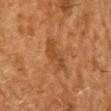The lesion was tiled from a total-body skin photograph and was not biopsied. Approximately 4.5 mm at its widest. A male patient aged around 70. The lesion is located on the head or neck. A 15 mm crop from a total-body photograph taken for skin-cancer surveillance. The tile uses cross-polarized illumination. Automated image analysis of the tile measured a lesion area of about 8.5 mm², an eccentricity of roughly 0.9, and a symmetry-axis asymmetry near 0.2. The software also gave border irregularity of about 3 on a 0–10 scale, internal color variation of about 3 on a 0–10 scale, and peripheral color asymmetry of about 1.